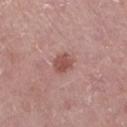Captured during whole-body skin photography for melanoma surveillance; the lesion was not biopsied. A female subject, in their mid-70s. Cropped from a whole-body photographic skin survey; the tile spans about 15 mm. The lesion is located on the left lower leg.